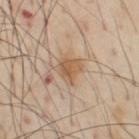The lesion was photographed on a routine skin check and not biopsied; there is no pathology result. A male subject aged 53 to 57. On the chest. Measured at roughly 3 mm in maximum diameter. Cropped from a total-body skin-imaging series; the visible field is about 15 mm. Captured under cross-polarized illumination.